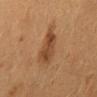Captured during whole-body skin photography for melanoma surveillance; the lesion was not biopsied.
A female patient aged around 50.
The recorded lesion diameter is about 4.5 mm.
The lesion is on the mid back.
A 15 mm crop from a total-body photograph taken for skin-cancer surveillance.
The total-body-photography lesion software estimated a lesion color around L≈36 a*≈18 b*≈29 in CIELAB, about 8 CIELAB-L* units darker than the surrounding skin, and a normalized border contrast of about 7.5. It also reported border irregularity of about 3 on a 0–10 scale, a within-lesion color-variation index near 3.5/10, and a peripheral color-asymmetry measure near 1.5. It also reported an automated nevus-likeness rating near 55 out of 100.